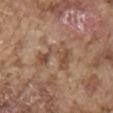Impression: Captured during whole-body skin photography for melanoma surveillance; the lesion was not biopsied. Background: A 15 mm crop from a total-body photograph taken for skin-cancer surveillance. A male patient, approximately 75 years of age. From the right upper arm.